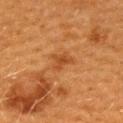This lesion was catalogued during total-body skin photography and was not selected for biopsy.
A region of skin cropped from a whole-body photographic capture, roughly 15 mm wide.
On the upper back.
This is a cross-polarized tile.
The patient is a female approximately 40 years of age.
The lesion's longest dimension is about 2.5 mm.